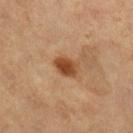Notes:
- workup: catalogued during a skin exam; not biopsied
- subject: female, aged approximately 70
- acquisition: ~15 mm crop, total-body skin-cancer survey
- lesion size: ~3 mm (longest diameter)
- location: the right lower leg
- lighting: cross-polarized
- automated metrics: an outline eccentricity of about 0.75 (0 = round, 1 = elongated) and a shape-asymmetry score of about 0.2 (0 = symmetric); a mean CIELAB color near L≈44 a*≈23 b*≈35 and a lesion–skin lightness drop of about 13; a border-irregularity rating of about 1.5/10, internal color variation of about 3 on a 0–10 scale, and a peripheral color-asymmetry measure near 1; a nevus-likeness score of about 95/100 and a lesion-detection confidence of about 100/100